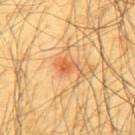Case summary:
- biopsy status: catalogued during a skin exam; not biopsied
- site: the mid back
- subject: male, about 65 years old
- tile lighting: cross-polarized illumination
- diameter: ~3.5 mm (longest diameter)
- image source: 15 mm crop, total-body photography